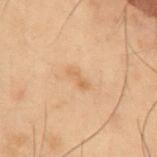size: about 2.5 mm; illumination: cross-polarized; anatomic site: the abdomen; patient: male, about 55 years old; imaging modality: ~15 mm tile from a whole-body skin photo.